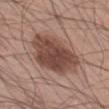Located on the left thigh.
This image is a 15 mm lesion crop taken from a total-body photograph.
A male patient aged approximately 60.